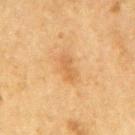| field | value |
|---|---|
| location | the arm |
| subject | male, roughly 65 years of age |
| illumination | cross-polarized |
| image-analysis metrics | roughly 6 lightness units darker than nearby skin and a lesion-to-skin contrast of about 5 (normalized; higher = more distinct) |
| imaging modality | 15 mm crop, total-body photography |
| lesion diameter | ~3.5 mm (longest diameter) |